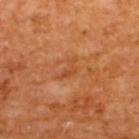| field | value |
|---|---|
| workup | no biopsy performed (imaged during a skin exam) |
| location | the upper back |
| subject | male, about 65 years old |
| tile lighting | cross-polarized illumination |
| imaging modality | ~15 mm tile from a whole-body skin photo |
| lesion size | about 3 mm |
| automated metrics | a footprint of about 3 mm², a shape eccentricity near 0.85, and a shape-asymmetry score of about 0.55 (0 = symmetric); a mean CIELAB color near L≈47 a*≈27 b*≈39 and a lesion-to-skin contrast of about 5 (normalized; higher = more distinct); a border-irregularity rating of about 6.5/10, a within-lesion color-variation index near 0/10, and radial color variation of about 0; a nevus-likeness score of about 0/100 and lesion-presence confidence of about 100/100 |